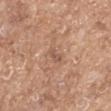The lesion was tiled from a total-body skin photograph and was not biopsied.
This is a white-light tile.
The lesion is on the left upper arm.
A male patient aged approximately 70.
Cropped from a whole-body photographic skin survey; the tile spans about 15 mm.